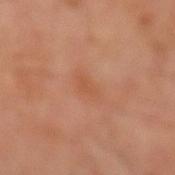notes = total-body-photography surveillance lesion; no biopsy | subject = male, aged approximately 50 | lesion diameter = ~2.5 mm (longest diameter) | acquisition = ~15 mm crop, total-body skin-cancer survey | lighting = cross-polarized illumination | body site = the left arm.